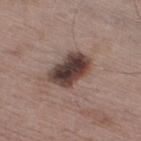Q: Automated lesion metrics?
A: a shape eccentricity near 0.75 and a shape-asymmetry score of about 0.2 (0 = symmetric); a mean CIELAB color near L≈39 a*≈16 b*≈20, roughly 17 lightness units darker than nearby skin, and a normalized border contrast of about 13.5; a border-irregularity rating of about 2/10, internal color variation of about 7 on a 0–10 scale, and a peripheral color-asymmetry measure near 2; a classifier nevus-likeness of about 40/100 and a detector confidence of about 100 out of 100 that the crop contains a lesion
Q: What is the anatomic site?
A: the right thigh
Q: How was this image acquired?
A: ~15 mm crop, total-body skin-cancer survey
Q: What is the lesion's diameter?
A: ≈5 mm
Q: Who is the patient?
A: male, aged around 65
Q: What lighting was used for the tile?
A: white-light